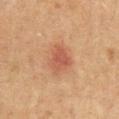biopsy_status: not biopsied; imaged during a skin examination
automated_metrics:
  cielab_L: 44
  cielab_a: 21
  cielab_b: 27
  border_irregularity_0_10: 2.5
  color_variation_0_10: 2.5
  peripheral_color_asymmetry: 1.0
  nevus_likeness_0_100: 75
  lesion_detection_confidence_0_100: 100
patient:
  sex: male
  age_approx: 50
lesion_size:
  long_diameter_mm_approx: 3.5
site: chest
lighting: cross-polarized
image:
  source: total-body photography crop
  field_of_view_mm: 15